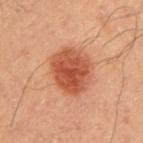No biopsy was performed on this lesion — it was imaged during a full skin examination and was not determined to be concerning.
A male subject roughly 40 years of age.
Imaged with cross-polarized lighting.
The lesion is located on the right upper arm.
A 15 mm crop from a total-body photograph taken for skin-cancer surveillance.